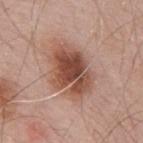Acquisition and patient details:
The subject is a male aged approximately 55. Measured at roughly 6.5 mm in maximum diameter. Cropped from a whole-body photographic skin survey; the tile spans about 15 mm. Imaged with white-light lighting. The total-body-photography lesion software estimated an area of roughly 21 mm², an eccentricity of roughly 0.75, and a symmetry-axis asymmetry near 0.2. The software also gave a lesion color around L≈50 a*≈22 b*≈28 in CIELAB, roughly 14 lightness units darker than nearby skin, and a lesion-to-skin contrast of about 10 (normalized; higher = more distinct). The lesion is on the mid back.